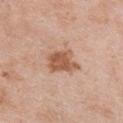Case summary:
– follow-up — catalogued during a skin exam; not biopsied
– imaging modality — ~15 mm tile from a whole-body skin photo
– automated lesion analysis — a lesion area of about 8.5 mm², an outline eccentricity of about 0.65 (0 = round, 1 = elongated), and a shape-asymmetry score of about 0.2 (0 = symmetric); a mean CIELAB color near L≈56 a*≈22 b*≈32, about 12 CIELAB-L* units darker than the surrounding skin, and a lesion-to-skin contrast of about 8.5 (normalized; higher = more distinct); a border-irregularity index near 3/10, a within-lesion color-variation index near 3/10, and peripheral color asymmetry of about 1
– lesion size — ≈4 mm
– lighting — white-light illumination
– patient — female, aged 48 to 52
– location — the chest